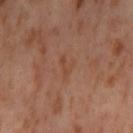biopsy status = total-body-photography surveillance lesion; no biopsy
body site = the left thigh
subject = female, about 55 years old
illumination = cross-polarized
imaging modality = ~15 mm crop, total-body skin-cancer survey